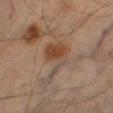Part of a total-body skin-imaging series; this lesion was reviewed on a skin check and was not flagged for biopsy.
A male patient aged around 65.
A 15 mm close-up tile from a total-body photography series done for melanoma screening.
On the left thigh.
The total-body-photography lesion software estimated an average lesion color of about L≈35 a*≈14 b*≈23 (CIELAB), about 7 CIELAB-L* units darker than the surrounding skin, and a normalized border contrast of about 7. It also reported a border-irregularity rating of about 8/10, a color-variation rating of about 2.5/10, and a peripheral color-asymmetry measure near 1. It also reported a detector confidence of about 95 out of 100 that the crop contains a lesion.